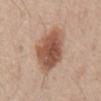Impression: Part of a total-body skin-imaging series; this lesion was reviewed on a skin check and was not flagged for biopsy. Background: On the mid back. Imaged with white-light lighting. Measured at roughly 6 mm in maximum diameter. The lesion-visualizer software estimated a detector confidence of about 100 out of 100 that the crop contains a lesion. A 15 mm close-up tile from a total-body photography series done for melanoma screening. The subject is a male aged 53–57.